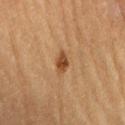biopsy status = imaged on a skin check; not biopsied | automated metrics = a mean CIELAB color near L≈40 a*≈20 b*≈33 and about 11 CIELAB-L* units darker than the surrounding skin; a border-irregularity index near 3/10, a within-lesion color-variation index near 3/10, and peripheral color asymmetry of about 1; a classifier nevus-likeness of about 95/100 and a detector confidence of about 100 out of 100 that the crop contains a lesion | size = about 2.5 mm | image source = total-body-photography crop, ~15 mm field of view | body site = the lower back | patient = male, aged around 85 or older.